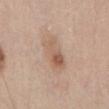Q: Was this lesion biopsied?
A: imaged on a skin check; not biopsied
Q: How was this image acquired?
A: total-body-photography crop, ~15 mm field of view
Q: Where on the body is the lesion?
A: the chest
Q: Lesion size?
A: ≈5 mm
Q: What did automated image analysis measure?
A: a lesion area of about 11 mm², an eccentricity of roughly 0.9, and a shape-asymmetry score of about 0.15 (0 = symmetric); a border-irregularity index near 2.5/10, a color-variation rating of about 7.5/10, and peripheral color asymmetry of about 3
Q: Illumination type?
A: white-light illumination
Q: Patient demographics?
A: male, in their 70s